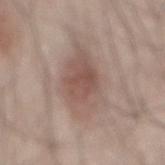follow-up=total-body-photography surveillance lesion; no biopsy | patient=male, in their mid-40s | lesion diameter=≈9.5 mm | automated lesion analysis=an eccentricity of roughly 0.9 and a symmetry-axis asymmetry near 0.2; a lesion color around L≈54 a*≈16 b*≈23 in CIELAB, about 9 CIELAB-L* units darker than the surrounding skin, and a normalized border contrast of about 6.5; a color-variation rating of about 5/10 and peripheral color asymmetry of about 1.5 | lighting=white-light illumination | body site=the mid back | image source=15 mm crop, total-body photography.